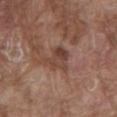Clinical impression: No biopsy was performed on this lesion — it was imaged during a full skin examination and was not determined to be concerning. Image and clinical context: Longest diameter approximately 3.5 mm. The lesion-visualizer software estimated border irregularity of about 4 on a 0–10 scale, a within-lesion color-variation index near 5/10, and radial color variation of about 1.5. The analysis additionally found an automated nevus-likeness rating near 0 out of 100 and a lesion-detection confidence of about 100/100. The lesion is located on the mid back. A male patient, aged 78 to 82. This is a white-light tile. A lesion tile, about 15 mm wide, cut from a 3D total-body photograph.